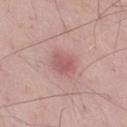- size: ≈2.5 mm
- image source: 15 mm crop, total-body photography
- site: the right thigh
- subject: male, aged around 55
- illumination: white-light illumination